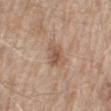patient=male, aged 78 to 82
illumination=white-light illumination
site=the mid back
image source=~15 mm tile from a whole-body skin photo
diameter=≈3.5 mm